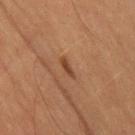Captured during whole-body skin photography for melanoma surveillance; the lesion was not biopsied. The lesion is located on the leg. A male subject about 60 years old. An algorithmic analysis of the crop reported a footprint of about 2.5 mm² and an outline eccentricity of about 0.95 (0 = round, 1 = elongated). And it measured a mean CIELAB color near L≈39 a*≈21 b*≈31, a lesion–skin lightness drop of about 8, and a normalized lesion–skin contrast near 7.5. The software also gave a border-irregularity rating of about 3/10, internal color variation of about 0 on a 0–10 scale, and a peripheral color-asymmetry measure near 0. The lesion's longest dimension is about 3 mm. A 15 mm close-up tile from a total-body photography series done for melanoma screening.